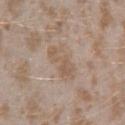Q: Was a biopsy performed?
A: total-body-photography surveillance lesion; no biopsy
Q: Where on the body is the lesion?
A: the left forearm
Q: Illumination type?
A: white-light illumination
Q: Patient demographics?
A: female, in their mid- to late 20s
Q: How was this image acquired?
A: ~15 mm crop, total-body skin-cancer survey